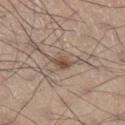<tbp_lesion>
  <biopsy_status>not biopsied; imaged during a skin examination</biopsy_status>
  <site>right lower leg</site>
  <patient>
    <sex>male</sex>
    <age_approx>55</age_approx>
  </patient>
  <image>
    <source>total-body photography crop</source>
    <field_of_view_mm>15</field_of_view_mm>
  </image>
</tbp_lesion>